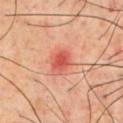<tbp_lesion>
<biopsy_status>not biopsied; imaged during a skin examination</biopsy_status>
<site>chest</site>
<image>
  <source>total-body photography crop</source>
  <field_of_view_mm>15</field_of_view_mm>
</image>
<patient>
  <sex>male</sex>
  <age_approx>70</age_approx>
</patient>
</tbp_lesion>